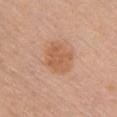Clinical impression:
This lesion was catalogued during total-body skin photography and was not selected for biopsy.
Clinical summary:
The patient is a female about 60 years old. The lesion is located on the chest. Cropped from a whole-body photographic skin survey; the tile spans about 15 mm.